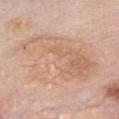biopsy status: catalogued during a skin exam; not biopsied | patient: female, about 60 years old | location: the chest | image source: ~15 mm crop, total-body skin-cancer survey.